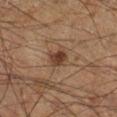biopsy status = catalogued during a skin exam; not biopsied
patient = male, in their 60s
acquisition = 15 mm crop, total-body photography
tile lighting = cross-polarized illumination
site = the right lower leg